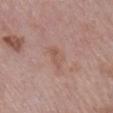  biopsy_status: not biopsied; imaged during a skin examination
  patient:
    sex: female
    age_approx: 70
  image:
    source: total-body photography crop
    field_of_view_mm: 15
  automated_metrics:
    area_mm2_approx: 4.5
    lesion_detection_confidence_0_100: 100
  lighting: white-light
  site: right lower leg